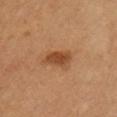Assessment:
Part of a total-body skin-imaging series; this lesion was reviewed on a skin check and was not flagged for biopsy.
Clinical summary:
Imaged with cross-polarized lighting. A male subject in their mid-50s. Automated tile analysis of the lesion measured roughly 10 lightness units darker than nearby skin and a lesion-to-skin contrast of about 8 (normalized; higher = more distinct). The software also gave a classifier nevus-likeness of about 95/100 and lesion-presence confidence of about 100/100. The recorded lesion diameter is about 3 mm. A 15 mm crop from a total-body photograph taken for skin-cancer surveillance. Located on the left upper arm.